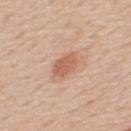Captured during whole-body skin photography for melanoma surveillance; the lesion was not biopsied. A close-up tile cropped from a whole-body skin photograph, about 15 mm across. A male patient aged 58 to 62. An algorithmic analysis of the crop reported an average lesion color of about L≈60 a*≈24 b*≈31 (CIELAB), roughly 10 lightness units darker than nearby skin, and a normalized border contrast of about 6.5. The analysis additionally found an automated nevus-likeness rating near 95 out of 100 and lesion-presence confidence of about 100/100. Longest diameter approximately 3 mm. The tile uses white-light illumination. The lesion is located on the upper back.